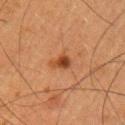Assessment:
Recorded during total-body skin imaging; not selected for excision or biopsy.
Clinical summary:
The lesion's longest dimension is about 2.5 mm. The patient is a male aged 48–52. Imaged with cross-polarized lighting. A lesion tile, about 15 mm wide, cut from a 3D total-body photograph. From the left upper arm.